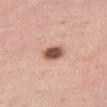No biopsy was performed on this lesion — it was imaged during a full skin examination and was not determined to be concerning. This is a white-light tile. The lesion is located on the right thigh. The recorded lesion diameter is about 3 mm. Automated tile analysis of the lesion measured a classifier nevus-likeness of about 100/100 and a detector confidence of about 100 out of 100 that the crop contains a lesion. A female patient in their mid- to late 40s. A close-up tile cropped from a whole-body skin photograph, about 15 mm across.